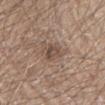Clinical impression:
The lesion was tiled from a total-body skin photograph and was not biopsied.
Background:
On the left forearm. A 15 mm close-up extracted from a 3D total-body photography capture. An algorithmic analysis of the crop reported a shape eccentricity near 0.65 and a shape-asymmetry score of about 0.2 (0 = symmetric). The software also gave about 8 CIELAB-L* units darker than the surrounding skin. The software also gave a nevus-likeness score of about 0/100 and lesion-presence confidence of about 100/100. About 3 mm across. The patient is a male roughly 65 years of age.